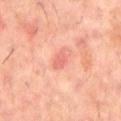| field | value |
|---|---|
| biopsy status | total-body-photography surveillance lesion; no biopsy |
| illumination | cross-polarized illumination |
| location | the mid back |
| automated metrics | an outline eccentricity of about 0.85 (0 = round, 1 = elongated) and two-axis asymmetry of about 0.2; a border-irregularity rating of about 2/10 and a peripheral color-asymmetry measure near 0; an automated nevus-likeness rating near 0 out of 100 and a detector confidence of about 100 out of 100 that the crop contains a lesion |
| lesion diameter | about 3 mm |
| subject | male, aged 58–62 |
| acquisition | ~15 mm tile from a whole-body skin photo |